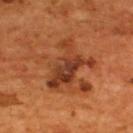Assessment: No biopsy was performed on this lesion — it was imaged during a full skin examination and was not determined to be concerning. Image and clinical context: A 15 mm close-up tile from a total-body photography series done for melanoma screening. The tile uses cross-polarized illumination. From the upper back. Longest diameter approximately 6 mm. A male patient aged 53–57.